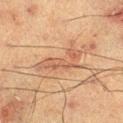{"biopsy_status": "not biopsied; imaged during a skin examination", "site": "leg", "automated_metrics": {"area_mm2_approx": 9.0, "eccentricity": 0.95, "shape_asymmetry": 0.45, "color_variation_0_10": 3.0, "peripheral_color_asymmetry": 1.0, "lesion_detection_confidence_0_100": 95}, "lesion_size": {"long_diameter_mm_approx": 6.0}, "patient": {"sex": "male", "age_approx": 60}, "lighting": "cross-polarized", "image": {"source": "total-body photography crop", "field_of_view_mm": 15}}